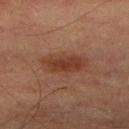Imaged with cross-polarized lighting. A roughly 15 mm field-of-view crop from a total-body skin photograph. The patient is a male aged 83 or over. On the right lower leg. The total-body-photography lesion software estimated a lesion color around L≈33 a*≈20 b*≈26 in CIELAB, a lesion–skin lightness drop of about 8, and a normalized border contrast of about 8. The analysis additionally found a border-irregularity rating of about 3/10, a color-variation rating of about 2.5/10, and a peripheral color-asymmetry measure near 1. Measured at roughly 5.5 mm in maximum diameter.